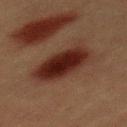lighting: cross-polarized
patient:
  sex: male
  age_approx: 40
site: mid back
image:
  source: total-body photography crop
  field_of_view_mm: 15
lesion_size:
  long_diameter_mm_approx: 7.0